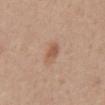<lesion>
<biopsy_status>not biopsied; imaged during a skin examination</biopsy_status>
<patient>
  <sex>male</sex>
  <age_approx>60</age_approx>
</patient>
<image>
  <source>total-body photography crop</source>
  <field_of_view_mm>15</field_of_view_mm>
</image>
<lighting>white-light</lighting>
<lesion_size>
  <long_diameter_mm_approx>2.5</long_diameter_mm_approx>
</lesion_size>
<site>abdomen</site>
</lesion>